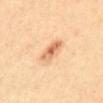Approximately 3 mm at its widest. Cropped from a whole-body photographic skin survey; the tile spans about 15 mm. From the abdomen. The total-body-photography lesion software estimated an eccentricity of roughly 0.85 and a symmetry-axis asymmetry near 0.25. And it measured a border-irregularity rating of about 2.5/10 and a color-variation rating of about 5/10. The analysis additionally found a nevus-likeness score of about 90/100. A male patient, in their 40s. The tile uses cross-polarized illumination.